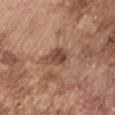follow-up — no biopsy performed (imaged during a skin exam)
image-analysis metrics — an area of roughly 6.5 mm² and an outline eccentricity of about 0.75 (0 = round, 1 = elongated); a lesion color around L≈47 a*≈20 b*≈28 in CIELAB, roughly 11 lightness units darker than nearby skin, and a normalized border contrast of about 8.5
lesion size — ≈3.5 mm
imaging modality — ~15 mm tile from a whole-body skin photo
tile lighting — white-light
subject — male, about 75 years old
site — the arm